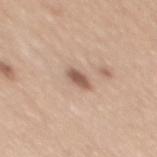Clinical impression:
The lesion was tiled from a total-body skin photograph and was not biopsied.
Context:
Cropped from a whole-body photographic skin survey; the tile spans about 15 mm. A female patient about 45 years old. Longest diameter approximately 3 mm. On the mid back.